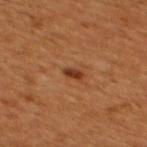Part of a total-body skin-imaging series; this lesion was reviewed on a skin check and was not flagged for biopsy. A male patient approximately 45 years of age. Approximately 2 mm at its widest. This is a cross-polarized tile. From the upper back. Cropped from a total-body skin-imaging series; the visible field is about 15 mm. An algorithmic analysis of the crop reported a footprint of about 2.5 mm², a shape eccentricity near 0.8, and a symmetry-axis asymmetry near 0.2. It also reported a border-irregularity rating of about 2/10, a within-lesion color-variation index near 2/10, and radial color variation of about 0.5. It also reported a nevus-likeness score of about 85/100 and a lesion-detection confidence of about 100/100.